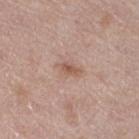size = about 3 mm
automated lesion analysis = a border-irregularity index near 2.5/10 and a peripheral color-asymmetry measure near 1; an automated nevus-likeness rating near 20 out of 100 and lesion-presence confidence of about 100/100
tile lighting = white-light illumination
imaging modality = ~15 mm crop, total-body skin-cancer survey
anatomic site = the right thigh
subject = female, aged around 40